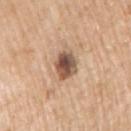Recorded during total-body skin imaging; not selected for excision or biopsy. The lesion is located on the right upper arm. A roughly 15 mm field-of-view crop from a total-body skin photograph. The total-body-photography lesion software estimated a lesion color around L≈53 a*≈18 b*≈29 in CIELAB, roughly 17 lightness units darker than nearby skin, and a normalized border contrast of about 11.5. And it measured border irregularity of about 1.5 on a 0–10 scale and radial color variation of about 2.5. This is a white-light tile. Longest diameter approximately 3.5 mm. The patient is a male aged 58–62.